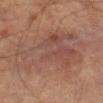  biopsy_status: not biopsied; imaged during a skin examination
  image:
    source: total-body photography crop
    field_of_view_mm: 15
  site: left thigh
  patient:
    sex: male
    age_approx: 65